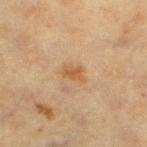Part of a total-body skin-imaging series; this lesion was reviewed on a skin check and was not flagged for biopsy.